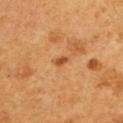{"biopsy_status": "not biopsied; imaged during a skin examination"}